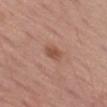follow-up: imaged on a skin check; not biopsied
site: the left thigh
image source: total-body-photography crop, ~15 mm field of view
lesion size: ~2.5 mm (longest diameter)
subject: male, aged 63 to 67